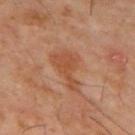Clinical summary: A male subject aged 58–62. A 15 mm crop from a total-body photograph taken for skin-cancer surveillance. On the mid back.